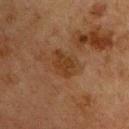Clinical impression:
Part of a total-body skin-imaging series; this lesion was reviewed on a skin check and was not flagged for biopsy.
Clinical summary:
Imaged with cross-polarized lighting. Located on the chest. About 3.5 mm across. Automated image analysis of the tile measured border irregularity of about 2.5 on a 0–10 scale and radial color variation of about 0.5. The software also gave a classifier nevus-likeness of about 0/100. A close-up tile cropped from a whole-body skin photograph, about 15 mm across. A male subject about 75 years old.